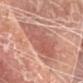follow-up: no biopsy performed (imaged during a skin exam); image source: ~15 mm tile from a whole-body skin photo; tile lighting: white-light illumination; patient: female, in their mid-70s; site: the head or neck.